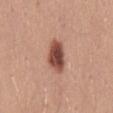No biopsy was performed on this lesion — it was imaged during a full skin examination and was not determined to be concerning. A 15 mm crop from a total-body photograph taken for skin-cancer surveillance. A male patient approximately 25 years of age. An algorithmic analysis of the crop reported a within-lesion color-variation index near 5/10 and radial color variation of about 1.5. About 4 mm across. From the mid back. This is a white-light tile.